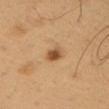• workup — total-body-photography surveillance lesion; no biopsy
• lighting — cross-polarized
• image — ~15 mm tile from a whole-body skin photo
• subject — male, in their 50s
• anatomic site — the right upper arm